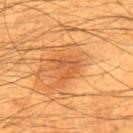<record>
<lesion_size>
  <long_diameter_mm_approx>3.0</long_diameter_mm_approx>
</lesion_size>
<automated_metrics>
  <vs_skin_contrast_norm>5.0</vs_skin_contrast_norm>
  <nevus_likeness_0_100>0</nevus_likeness_0_100>
  <lesion_detection_confidence_0_100>100</lesion_detection_confidence_0_100>
</automated_metrics>
<image>
  <source>total-body photography crop</source>
  <field_of_view_mm>15</field_of_view_mm>
</image>
<site>back</site>
<patient>
  <sex>male</sex>
  <age_approx>60</age_approx>
</patient>
</record>